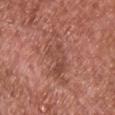<tbp_lesion>
  <automated_metrics>
    <area_mm2_approx>11.0</area_mm2_approx>
    <eccentricity>0.9</eccentricity>
    <shape_asymmetry>0.45</shape_asymmetry>
    <cielab_L>48</cielab_L>
    <cielab_a>25</cielab_a>
    <cielab_b>28</cielab_b>
    <border_irregularity_0_10>7.5</border_irregularity_0_10>
    <color_variation_0_10>4.5</color_variation_0_10>
    <nevus_likeness_0_100>0</nevus_likeness_0_100>
    <lesion_detection_confidence_0_100>100</lesion_detection_confidence_0_100>
  </automated_metrics>
  <site>chest</site>
  <lighting>white-light</lighting>
  <patient>
    <sex>male</sex>
    <age_approx>65</age_approx>
  </patient>
  <image>
    <source>total-body photography crop</source>
    <field_of_view_mm>15</field_of_view_mm>
  </image>
</tbp_lesion>